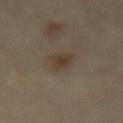workup: total-body-photography surveillance lesion; no biopsy
subject: female, roughly 60 years of age
imaging modality: total-body-photography crop, ~15 mm field of view
size: ≈3.5 mm
anatomic site: the back
illumination: cross-polarized illumination